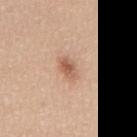Assessment: Imaged during a routine full-body skin examination; the lesion was not biopsied and no histopathology is available. Acquisition and patient details: A male subject, in their 40s. This image is a 15 mm lesion crop taken from a total-body photograph. Automated image analysis of the tile measured an area of roughly 4.5 mm² and two-axis asymmetry of about 0.25. On the mid back.